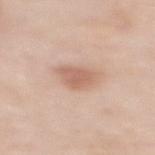biopsy status: no biopsy performed (imaged during a skin exam)
anatomic site: the back
patient: female, aged around 50
image source: 15 mm crop, total-body photography
diameter: about 3.5 mm